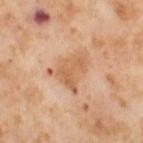Part of a total-body skin-imaging series; this lesion was reviewed on a skin check and was not flagged for biopsy.